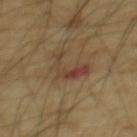biopsy status — total-body-photography surveillance lesion; no biopsy | automated metrics — a footprint of about 8 mm², an outline eccentricity of about 0.8 (0 = round, 1 = elongated), and a symmetry-axis asymmetry near 0.7; a nevus-likeness score of about 0/100 and a lesion-detection confidence of about 100/100 | tile lighting — cross-polarized illumination | patient — male, approximately 65 years of age | image source — ~15 mm crop, total-body skin-cancer survey | anatomic site — the mid back | size — ~5 mm (longest diameter).